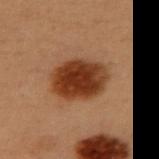Q: Was a biopsy performed?
A: imaged on a skin check; not biopsied
Q: Where on the body is the lesion?
A: the back
Q: Who is the patient?
A: female, aged approximately 50
Q: Illumination type?
A: cross-polarized
Q: What kind of image is this?
A: ~15 mm tile from a whole-body skin photo
Q: What did automated image analysis measure?
A: a footprint of about 18 mm², a shape eccentricity near 0.7, and two-axis asymmetry of about 0.1; an average lesion color of about L≈31 a*≈22 b*≈30 (CIELAB) and a lesion–skin lightness drop of about 15; a border-irregularity index near 1.5/10, internal color variation of about 4.5 on a 0–10 scale, and radial color variation of about 1.5
Q: How large is the lesion?
A: about 5.5 mm